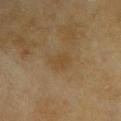follow-up = catalogued during a skin exam; not biopsied
image source = 15 mm crop, total-body photography
body site = the upper back
lesion size = ≈2.5 mm
patient = female, about 60 years old
image-analysis metrics = an average lesion color of about L≈41 a*≈14 b*≈32 (CIELAB), roughly 5 lightness units darker than nearby skin, and a normalized lesion–skin contrast near 5; a border-irregularity rating of about 3/10 and radial color variation of about 0.5